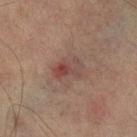Q: Is there a histopathology result?
A: imaged on a skin check; not biopsied
Q: What is the imaging modality?
A: ~15 mm crop, total-body skin-cancer survey
Q: What lighting was used for the tile?
A: cross-polarized illumination
Q: Who is the patient?
A: male, in their mid-70s
Q: What is the anatomic site?
A: the right lower leg